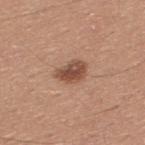<tbp_lesion>
  <biopsy_status>not biopsied; imaged during a skin examination</biopsy_status>
</tbp_lesion>